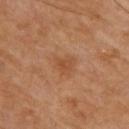| key | value |
|---|---|
| biopsy status | no biopsy performed (imaged during a skin exam) |
| illumination | cross-polarized illumination |
| subject | male, aged 68–72 |
| lesion diameter | ~2.5 mm (longest diameter) |
| body site | the upper back |
| image source | ~15 mm tile from a whole-body skin photo |
| automated lesion analysis | a shape eccentricity near 0.25; a mean CIELAB color near L≈48 a*≈22 b*≈34 and a normalized border contrast of about 5.5; a detector confidence of about 100 out of 100 that the crop contains a lesion |